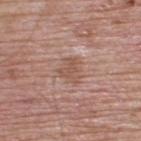Findings:
– biopsy status: total-body-photography surveillance lesion; no biopsy
– site: the upper back
– illumination: white-light
– patient: male, approximately 65 years of age
– automated metrics: a border-irregularity index near 3/10, internal color variation of about 1.5 on a 0–10 scale, and peripheral color asymmetry of about 0.5; an automated nevus-likeness rating near 0 out of 100 and a detector confidence of about 100 out of 100 that the crop contains a lesion
– image: 15 mm crop, total-body photography
– lesion diameter: ~3 mm (longest diameter)